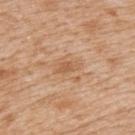  biopsy_status: not biopsied; imaged during a skin examination
  lighting: white-light
  site: back
  image:
    source: total-body photography crop
    field_of_view_mm: 15
  patient:
    sex: male
    age_approx: 65
  automated_metrics:
    area_mm2_approx: 3.0
    eccentricity: 0.85
    shape_asymmetry: 0.4
    cielab_L: 58
    cielab_a: 21
    cielab_b: 36
    vs_skin_darker_L: 8.0
    vs_skin_contrast_norm: 6.0
    nevus_likeness_0_100: 0
    lesion_detection_confidence_0_100: 100